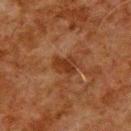follow-up: catalogued during a skin exam; not biopsied
site: the upper back
size: ~3 mm (longest diameter)
patient: male, aged around 80
image: ~15 mm crop, total-body skin-cancer survey
automated metrics: roughly 7 lightness units darker than nearby skin and a lesion-to-skin contrast of about 7.5 (normalized; higher = more distinct)